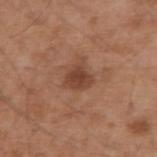Clinical impression:
No biopsy was performed on this lesion — it was imaged during a full skin examination and was not determined to be concerning.
Image and clinical context:
On the right upper arm. A male patient, about 55 years old. A region of skin cropped from a whole-body photographic capture, roughly 15 mm wide.